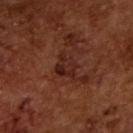Impression:
Recorded during total-body skin imaging; not selected for excision or biopsy.
Image and clinical context:
A male patient, approximately 65 years of age. A 15 mm close-up extracted from a 3D total-body photography capture. Imaged with cross-polarized lighting.